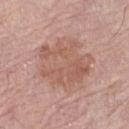Impression:
Captured during whole-body skin photography for melanoma surveillance; the lesion was not biopsied.
Context:
A female patient, aged around 65. The lesion is on the left thigh. An algorithmic analysis of the crop reported a lesion–skin lightness drop of about 8. The software also gave border irregularity of about 3.5 on a 0–10 scale, internal color variation of about 4 on a 0–10 scale, and a peripheral color-asymmetry measure near 1.5. The analysis additionally found a nevus-likeness score of about 5/100 and lesion-presence confidence of about 100/100. Longest diameter approximately 6.5 mm. The tile uses white-light illumination. A roughly 15 mm field-of-view crop from a total-body skin photograph.